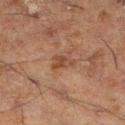{
  "biopsy_status": "not biopsied; imaged during a skin examination"
}